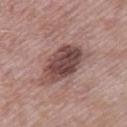{"biopsy_status": "not biopsied; imaged during a skin examination", "automated_metrics": {"area_mm2_approx": 17.0, "shape_asymmetry": 0.2, "color_variation_0_10": 5.5, "peripheral_color_asymmetry": 2.0, "lesion_detection_confidence_0_100": 100}, "site": "leg", "image": {"source": "total-body photography crop", "field_of_view_mm": 15}, "lighting": "white-light", "patient": {"sex": "male", "age_approx": 55}}